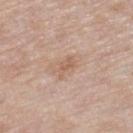Assessment:
Captured during whole-body skin photography for melanoma surveillance; the lesion was not biopsied.
Clinical summary:
On the right lower leg. An algorithmic analysis of the crop reported a lesion area of about 3 mm² and an eccentricity of roughly 0.9. Longest diameter approximately 3 mm. A female subject, aged around 60. A close-up tile cropped from a whole-body skin photograph, about 15 mm across. Imaged with white-light lighting.